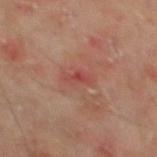biopsy_status: not biopsied; imaged during a skin examination
image:
  source: total-body photography crop
  field_of_view_mm: 15
patient:
  sex: male
  age_approx: 65
lesion_size:
  long_diameter_mm_approx: 2.5
site: mid back